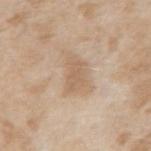Assessment:
Part of a total-body skin-imaging series; this lesion was reviewed on a skin check and was not flagged for biopsy.
Image and clinical context:
Automated image analysis of the tile measured a lesion area of about 5.5 mm², an eccentricity of roughly 0.85, and a symmetry-axis asymmetry near 0.3. Measured at roughly 3.5 mm in maximum diameter. The lesion is located on the right upper arm. A male subject, roughly 45 years of age. Cropped from a total-body skin-imaging series; the visible field is about 15 mm.